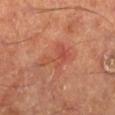A 15 mm crop from a total-body photograph taken for skin-cancer surveillance. A male subject approximately 65 years of age. The lesion is located on the left lower leg.